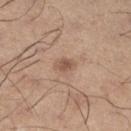Imaged during a routine full-body skin examination; the lesion was not biopsied and no histopathology is available.
A lesion tile, about 15 mm wide, cut from a 3D total-body photograph.
A male patient, in their mid- to late 50s.
This is a white-light tile.
From the leg.
Approximately 2.5 mm at its widest.